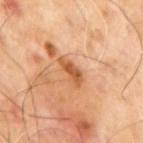Assessment:
This lesion was catalogued during total-body skin photography and was not selected for biopsy.
Image and clinical context:
From the mid back. A male patient in their mid- to late 60s. A 15 mm crop from a total-body photograph taken for skin-cancer surveillance.